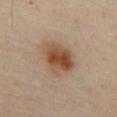biopsy status — imaged on a skin check; not biopsied
subject — male, roughly 55 years of age
image source — total-body-photography crop, ~15 mm field of view
site — the abdomen
lighting — cross-polarized
lesion size — ~4.5 mm (longest diameter)